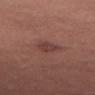Impression: The lesion was photographed on a routine skin check and not biopsied; there is no pathology result. Clinical summary: Located on the abdomen. The lesion-visualizer software estimated a footprint of about 5 mm², an eccentricity of roughly 0.65, and a shape-asymmetry score of about 0.2 (0 = symmetric). It also reported a mean CIELAB color near L≈40 a*≈23 b*≈21, about 7 CIELAB-L* units darker than the surrounding skin, and a lesion-to-skin contrast of about 6.5 (normalized; higher = more distinct). And it measured an automated nevus-likeness rating near 10 out of 100 and lesion-presence confidence of about 100/100. A female patient, roughly 30 years of age. This image is a 15 mm lesion crop taken from a total-body photograph. Measured at roughly 3 mm in maximum diameter.